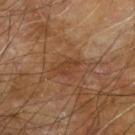Clinical summary:
The lesion is on the arm. The lesion's longest dimension is about 2.5 mm. This is a cross-polarized tile. The patient is a male aged 63 to 67. The lesion-visualizer software estimated a lesion color around L≈40 a*≈21 b*≈32 in CIELAB, a lesion–skin lightness drop of about 7, and a normalized lesion–skin contrast near 5.5. And it measured a border-irregularity index near 3/10, a within-lesion color-variation index near 1.5/10, and a peripheral color-asymmetry measure near 0.5. A roughly 15 mm field-of-view crop from a total-body skin photograph.